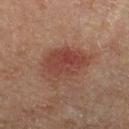body site = the right thigh
illumination = cross-polarized illumination
automated metrics = about 8 CIELAB-L* units darker than the surrounding skin and a normalized lesion–skin contrast near 7; border irregularity of about 2.5 on a 0–10 scale; an automated nevus-likeness rating near 90 out of 100 and a detector confidence of about 100 out of 100 that the crop contains a lesion
image source = ~15 mm crop, total-body skin-cancer survey
patient = male, roughly 65 years of age